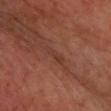Captured during whole-body skin photography for melanoma surveillance; the lesion was not biopsied.
Automated tile analysis of the lesion measured a lesion color around L≈35 a*≈22 b*≈27 in CIELAB and a lesion-to-skin contrast of about 5 (normalized; higher = more distinct). And it measured a border-irregularity index near 4/10 and radial color variation of about 0. And it measured an automated nevus-likeness rating near 0 out of 100 and lesion-presence confidence of about 75/100.
A male subject, approximately 60 years of age.
The lesion's longest dimension is about 2.5 mm.
This is a cross-polarized tile.
The lesion is on the upper back.
A 15 mm close-up tile from a total-body photography series done for melanoma screening.